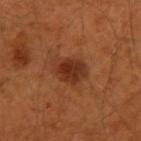Impression:
The lesion was photographed on a routine skin check and not biopsied; there is no pathology result.
Background:
The lesion is on the left arm. This is a cross-polarized tile. Automated tile analysis of the lesion measured an area of roughly 8.5 mm², a shape eccentricity near 0.65, and a symmetry-axis asymmetry near 0.15. And it measured a lesion–skin lightness drop of about 10. A male patient, roughly 50 years of age. About 4 mm across. A lesion tile, about 15 mm wide, cut from a 3D total-body photograph.